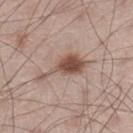Q: Is there a histopathology result?
A: imaged on a skin check; not biopsied
Q: Lesion size?
A: ≈6.5 mm
Q: Where on the body is the lesion?
A: the left lower leg
Q: What kind of image is this?
A: ~15 mm tile from a whole-body skin photo
Q: Automated lesion metrics?
A: about 13 CIELAB-L* units darker than the surrounding skin and a normalized lesion–skin contrast near 9.5; an automated nevus-likeness rating near 95 out of 100 and a detector confidence of about 100 out of 100 that the crop contains a lesion
Q: Patient demographics?
A: male, aged around 60
Q: What lighting was used for the tile?
A: white-light illumination